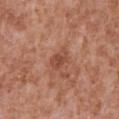Q: Was this lesion biopsied?
A: imaged on a skin check; not biopsied
Q: What kind of image is this?
A: total-body-photography crop, ~15 mm field of view
Q: What are the patient's age and sex?
A: male, aged around 45
Q: Lesion location?
A: the chest